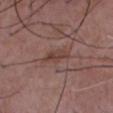biopsy_status: not biopsied; imaged during a skin examination
site: chest
patient:
  sex: male
  age_approx: 50
image:
  source: total-body photography crop
  field_of_view_mm: 15